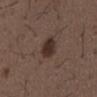This lesion was catalogued during total-body skin photography and was not selected for biopsy. The subject is a male aged 48 to 52. The lesion is located on the mid back. Cropped from a total-body skin-imaging series; the visible field is about 15 mm. The lesion's longest dimension is about 3.5 mm. The lesion-visualizer software estimated a mean CIELAB color near L≈30 a*≈15 b*≈20, about 11 CIELAB-L* units darker than the surrounding skin, and a lesion-to-skin contrast of about 10.5 (normalized; higher = more distinct). Imaged with white-light lighting.